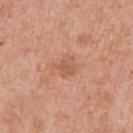Case summary:
– follow-up · catalogued during a skin exam; not biopsied
– subject · female, aged approximately 60
– image source · ~15 mm crop, total-body skin-cancer survey
– anatomic site · the right upper arm